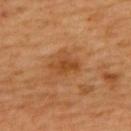– acquisition · ~15 mm tile from a whole-body skin photo
– tile lighting · cross-polarized illumination
– lesion diameter · about 3.5 mm
– patient · female, about 45 years old
– anatomic site · the upper back